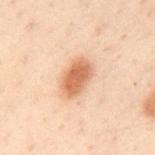| field | value |
|---|---|
| biopsy status | catalogued during a skin exam; not biopsied |
| subject | male, in their 50s |
| automated metrics | an eccentricity of roughly 0.8 and a symmetry-axis asymmetry near 0.2; a border-irregularity index near 2/10, internal color variation of about 3 on a 0–10 scale, and a peripheral color-asymmetry measure near 1; a classifier nevus-likeness of about 100/100 and a lesion-detection confidence of about 100/100 |
| lighting | cross-polarized |
| acquisition | ~15 mm tile from a whole-body skin photo |
| lesion diameter | about 4 mm |
| body site | the mid back |